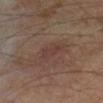* notes · no biopsy performed (imaged during a skin exam)
* anatomic site · the right lower leg
* subject · male, aged 63 to 67
* imaging modality · total-body-photography crop, ~15 mm field of view